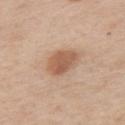The lesion was tiled from a total-body skin photograph and was not biopsied. A region of skin cropped from a whole-body photographic capture, roughly 15 mm wide. On the left upper arm. A male subject, aged approximately 60.